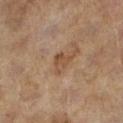Context:
A close-up tile cropped from a whole-body skin photograph, about 15 mm across. This is a cross-polarized tile. On the left lower leg. Approximately 3 mm at its widest. The subject is a female roughly 60 years of age.